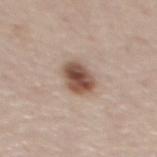Image and clinical context:
The lesion-visualizer software estimated a footprint of about 8.5 mm², an eccentricity of roughly 0.45, and a symmetry-axis asymmetry near 0.2. The analysis additionally found border irregularity of about 2 on a 0–10 scale and a peripheral color-asymmetry measure near 2. The analysis additionally found an automated nevus-likeness rating near 95 out of 100 and a detector confidence of about 100 out of 100 that the crop contains a lesion. The tile uses white-light illumination. Measured at roughly 3.5 mm in maximum diameter. The lesion is on the mid back. A close-up tile cropped from a whole-body skin photograph, about 15 mm across. A male subject, in their mid- to late 40s.